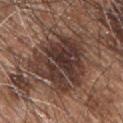The lesion was tiled from a total-body skin photograph and was not biopsied. Imaged with white-light lighting. Longest diameter approximately 8 mm. A lesion tile, about 15 mm wide, cut from a 3D total-body photograph. The lesion is located on the chest. A male subject, in their mid- to late 40s.